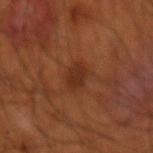No biopsy was performed on this lesion — it was imaged during a full skin examination and was not determined to be concerning.
Longest diameter approximately 2.5 mm.
This is a cross-polarized tile.
The lesion is on the right forearm.
The subject is a male in their mid- to late 50s.
Automated tile analysis of the lesion measured a mean CIELAB color near L≈30 a*≈24 b*≈30, a lesion–skin lightness drop of about 7, and a lesion-to-skin contrast of about 7 (normalized; higher = more distinct).
Cropped from a total-body skin-imaging series; the visible field is about 15 mm.